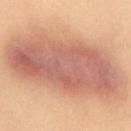Assessment:
No biopsy was performed on this lesion — it was imaged during a full skin examination and was not determined to be concerning.
Image and clinical context:
Approximately 16 mm at its widest. From the abdomen. A female subject, aged around 40. Imaged with cross-polarized lighting. This image is a 15 mm lesion crop taken from a total-body photograph.